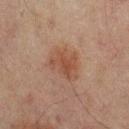{
  "biopsy_status": "not biopsied; imaged during a skin examination",
  "image": {
    "source": "total-body photography crop",
    "field_of_view_mm": 15
  },
  "site": "mid back",
  "lighting": "cross-polarized",
  "lesion_size": {
    "long_diameter_mm_approx": 4.0
  },
  "automated_metrics": {
    "shape_asymmetry": 0.3,
    "border_irregularity_0_10": 3.5,
    "color_variation_0_10": 2.5,
    "peripheral_color_asymmetry": 0.5
  },
  "patient": {
    "sex": "male",
    "age_approx": 65
  }
}